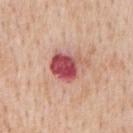The lesion was photographed on a routine skin check and not biopsied; there is no pathology result. A roughly 15 mm field-of-view crop from a total-body skin photograph. The patient is a male roughly 65 years of age. The lesion is on the back. Approximately 4.5 mm at its widest.